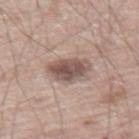Notes:
– biopsy status: catalogued during a skin exam; not biopsied
– lesion size: ≈4 mm
– image: total-body-photography crop, ~15 mm field of view
– lighting: white-light
– subject: male, aged approximately 70
– TBP lesion metrics: a classifier nevus-likeness of about 60/100
– body site: the left thigh